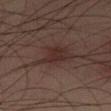| field | value |
|---|---|
| biopsy status | imaged on a skin check; not biopsied |
| location | the leg |
| lighting | cross-polarized illumination |
| automated lesion analysis | an average lesion color of about L≈26 a*≈14 b*≈17 (CIELAB) and a lesion–skin lightness drop of about 6; a border-irregularity rating of about 4.5/10, a color-variation rating of about 3/10, and peripheral color asymmetry of about 1 |
| patient | male, in their mid- to late 30s |
| image | ~15 mm crop, total-body skin-cancer survey |